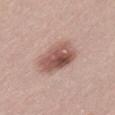The lesion was photographed on a routine skin check and not biopsied; there is no pathology result.
A 15 mm close-up tile from a total-body photography series done for melanoma screening.
The lesion-visualizer software estimated a shape eccentricity near 0.6 and a shape-asymmetry score of about 0.15 (0 = symmetric). The analysis additionally found a border-irregularity rating of about 2/10. The analysis additionally found a classifier nevus-likeness of about 90/100 and a detector confidence of about 100 out of 100 that the crop contains a lesion.
A female patient, about 65 years old.
The lesion's longest dimension is about 5 mm.
The lesion is on the left thigh.
The tile uses white-light illumination.